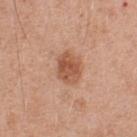No biopsy was performed on this lesion — it was imaged during a full skin examination and was not determined to be concerning. The lesion is located on the chest. Automated tile analysis of the lesion measured an area of roughly 8 mm². And it measured a border-irregularity rating of about 1.5/10, a color-variation rating of about 4/10, and radial color variation of about 1.5. Cropped from a whole-body photographic skin survey; the tile spans about 15 mm. Measured at roughly 3.5 mm in maximum diameter. The tile uses white-light illumination. The subject is a male aged approximately 55.